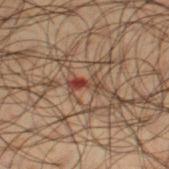Clinical impression:
Imaged during a routine full-body skin examination; the lesion was not biopsied and no histopathology is available.
Image and clinical context:
The patient is a male aged 48–52. The lesion is located on the left thigh. The tile uses cross-polarized illumination. A region of skin cropped from a whole-body photographic capture, roughly 15 mm wide.